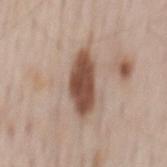Notes:
* follow-up: total-body-photography surveillance lesion; no biopsy
* imaging modality: ~15 mm tile from a whole-body skin photo
* automated lesion analysis: a border-irregularity rating of about 2.5/10, internal color variation of about 3 on a 0–10 scale, and a peripheral color-asymmetry measure near 1
* illumination: white-light illumination
* body site: the mid back
* subject: male, aged 63 to 67